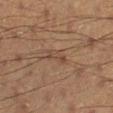biopsy_status: not biopsied; imaged during a skin examination
patient:
  sex: male
  age_approx: 55
site: right lower leg
automated_metrics:
  border_irregularity_0_10: 3.5
  color_variation_0_10: 2.0
  peripheral_color_asymmetry: 0.5
lighting: cross-polarized
lesion_size:
  long_diameter_mm_approx: 3.5
image:
  source: total-body photography crop
  field_of_view_mm: 15